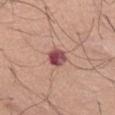{"biopsy_status": "not biopsied; imaged during a skin examination", "patient": {"sex": "male", "age_approx": 55}, "automated_metrics": {"eccentricity": 0.5, "border_irregularity_0_10": 1.5, "peripheral_color_asymmetry": 2.0, "nevus_likeness_0_100": 0, "lesion_detection_confidence_0_100": 100}, "lesion_size": {"long_diameter_mm_approx": 2.5}, "image": {"source": "total-body photography crop", "field_of_view_mm": 15}}